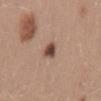<case>
<lesion_size>
  <long_diameter_mm_approx>2.5</long_diameter_mm_approx>
</lesion_size>
<patient>
  <sex>female</sex>
  <age_approx>25</age_approx>
</patient>
<lighting>white-light</lighting>
<site>mid back</site>
<image>
  <source>total-body photography crop</source>
  <field_of_view_mm>15</field_of_view_mm>
</image>
</case>